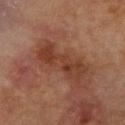This lesion was catalogued during total-body skin photography and was not selected for biopsy. The lesion is located on the left forearm. A female subject, approximately 80 years of age. A 15 mm crop from a total-body photograph taken for skin-cancer surveillance. Imaged with cross-polarized lighting.